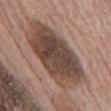Assessment: The lesion was tiled from a total-body skin photograph and was not biopsied. Clinical summary: The patient is a male aged 68 to 72. Imaged with white-light lighting. From the abdomen. Longest diameter approximately 11 mm. Cropped from a total-body skin-imaging series; the visible field is about 15 mm.